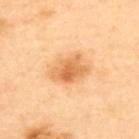The lesion was tiled from a total-body skin photograph and was not biopsied. This is a cross-polarized tile. The subject is a female roughly 40 years of age. The lesion is on the back. An algorithmic analysis of the crop reported a mean CIELAB color near L≈71 a*≈25 b*≈46, a lesion–skin lightness drop of about 12, and a normalized lesion–skin contrast near 7.5. And it measured a border-irregularity index near 2/10, internal color variation of about 5 on a 0–10 scale, and peripheral color asymmetry of about 1.5. The recorded lesion diameter is about 4.5 mm. A roughly 15 mm field-of-view crop from a total-body skin photograph.